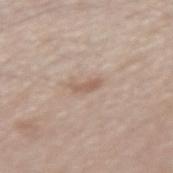Clinical impression:
Recorded during total-body skin imaging; not selected for excision or biopsy.
Context:
On the right forearm. A 15 mm close-up tile from a total-body photography series done for melanoma screening. The subject is a male aged 58 to 62.